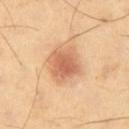Impression:
The lesion was tiled from a total-body skin photograph and was not biopsied.
Image and clinical context:
Located on the left thigh. The patient is a female aged 48–52. Longest diameter approximately 4.5 mm. The lesion-visualizer software estimated an outline eccentricity of about 0.6 (0 = round, 1 = elongated). The analysis additionally found a border-irregularity rating of about 2/10, internal color variation of about 4.5 on a 0–10 scale, and a peripheral color-asymmetry measure near 1. Cropped from a total-body skin-imaging series; the visible field is about 15 mm. Imaged with cross-polarized lighting.